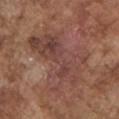The lesion was tiled from a total-body skin photograph and was not biopsied. A male subject, approximately 75 years of age. Cropped from a total-body skin-imaging series; the visible field is about 15 mm. Located on the left upper arm.